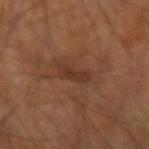notes=imaged on a skin check; not biopsied | automated lesion analysis=a mean CIELAB color near L≈33 a*≈19 b*≈28; a detector confidence of about 100 out of 100 that the crop contains a lesion | diameter=about 3 mm | patient=male, aged 58 to 62 | imaging modality=15 mm crop, total-body photography | illumination=cross-polarized illumination | site=the right forearm.